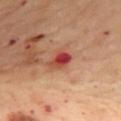- follow-up — catalogued during a skin exam; not biopsied
- subject — female, aged approximately 55
- acquisition — ~15 mm crop, total-body skin-cancer survey
- anatomic site — the mid back